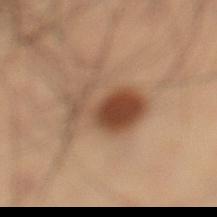Case summary:
* notes — no biopsy performed (imaged during a skin exam)
* image source — 15 mm crop, total-body photography
* site — the left lower leg
* subject — male, approximately 55 years of age
* image-analysis metrics — a mean CIELAB color near L≈37 a*≈16 b*≈26, roughly 11 lightness units darker than nearby skin, and a normalized lesion–skin contrast near 9.5; a border-irregularity rating of about 6/10 and a peripheral color-asymmetry measure near 1.5
* lesion diameter — about 6.5 mm
* lighting — cross-polarized